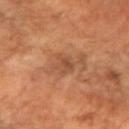follow-up — total-body-photography surveillance lesion; no biopsy | automated lesion analysis — a lesion area of about 5 mm² and an eccentricity of roughly 0.6 | tile lighting — cross-polarized | size — ~3 mm (longest diameter) | body site — the arm | acquisition — total-body-photography crop, ~15 mm field of view | subject — female, about 70 years old.